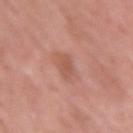• biopsy status: imaged on a skin check; not biopsied
• automated metrics: a footprint of about 3.5 mm², an eccentricity of roughly 0.75, and two-axis asymmetry of about 0.25; a lesion–skin lightness drop of about 8 and a normalized lesion–skin contrast near 5.5; border irregularity of about 2.5 on a 0–10 scale and peripheral color asymmetry of about 0.5; a nevus-likeness score of about 0/100
• lesion size: ≈2.5 mm
• subject: female, aged around 60
• imaging modality: ~15 mm crop, total-body skin-cancer survey
• anatomic site: the right upper arm